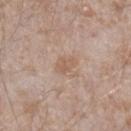Assessment:
Recorded during total-body skin imaging; not selected for excision or biopsy.
Image and clinical context:
The lesion is on the leg. Cropped from a total-body skin-imaging series; the visible field is about 15 mm. The subject is a male aged around 45.